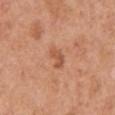The lesion was photographed on a routine skin check and not biopsied; there is no pathology result. The lesion is on the left upper arm. A female subject, aged around 40. A 15 mm close-up tile from a total-body photography series done for melanoma screening. Imaged with white-light lighting. The total-body-photography lesion software estimated a lesion area of about 3.5 mm², a shape eccentricity near 0.85, and two-axis asymmetry of about 0.45. And it measured a within-lesion color-variation index near 0/10.